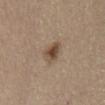Clinical impression:
Recorded during total-body skin imaging; not selected for excision or biopsy.
Acquisition and patient details:
The recorded lesion diameter is about 2.5 mm. The lesion is located on the front of the torso. A female patient, approximately 65 years of age. A roughly 15 mm field-of-view crop from a total-body skin photograph. The tile uses white-light illumination.